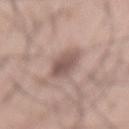| feature | finding |
|---|---|
| workup | no biopsy performed (imaged during a skin exam) |
| acquisition | total-body-photography crop, ~15 mm field of view |
| patient | male, aged approximately 70 |
| location | the mid back |